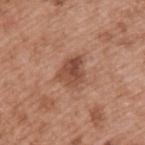Clinical impression:
The lesion was photographed on a routine skin check and not biopsied; there is no pathology result.
Clinical summary:
A male subject, in their mid- to late 50s. The lesion is on the upper back. A roughly 15 mm field-of-view crop from a total-body skin photograph. Measured at roughly 3.5 mm in maximum diameter.